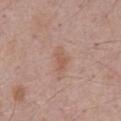Q: How was the tile lit?
A: white-light
Q: What is the anatomic site?
A: the front of the torso
Q: What is the lesion's diameter?
A: about 3.5 mm
Q: What is the imaging modality?
A: ~15 mm crop, total-body skin-cancer survey
Q: Patient demographics?
A: male, roughly 70 years of age
Q: Automated lesion metrics?
A: a lesion area of about 5 mm² and a symmetry-axis asymmetry near 0.4; a border-irregularity rating of about 4/10, internal color variation of about 1.5 on a 0–10 scale, and a peripheral color-asymmetry measure near 0.5; a classifier nevus-likeness of about 0/100